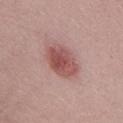Part of a total-body skin-imaging series; this lesion was reviewed on a skin check and was not flagged for biopsy. Cropped from a total-body skin-imaging series; the visible field is about 15 mm. The total-body-photography lesion software estimated a classifier nevus-likeness of about 95/100 and a lesion-detection confidence of about 100/100. Measured at roughly 5 mm in maximum diameter. A male subject about 50 years old. On the abdomen. This is a white-light tile.